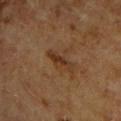Recorded during total-body skin imaging; not selected for excision or biopsy. This is a cross-polarized tile. The patient is a male aged 63–67. Located on the upper back. A 15 mm close-up extracted from a 3D total-body photography capture. About 4.5 mm across.